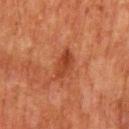workup: catalogued during a skin exam; not biopsied
image-analysis metrics: an average lesion color of about L≈34 a*≈25 b*≈30 (CIELAB), a lesion–skin lightness drop of about 8, and a lesion-to-skin contrast of about 7 (normalized; higher = more distinct); border irregularity of about 2.5 on a 0–10 scale, a within-lesion color-variation index near 4/10, and peripheral color asymmetry of about 1.5; an automated nevus-likeness rating near 5 out of 100 and lesion-presence confidence of about 100/100
image: 15 mm crop, total-body photography
body site: the mid back
subject: male, about 80 years old